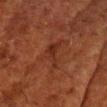| feature | finding |
|---|---|
| biopsy status | no biopsy performed (imaged during a skin exam) |
| diameter | ≈4.5 mm |
| site | the head or neck |
| patient | male, aged approximately 60 |
| image | ~15 mm tile from a whole-body skin photo |
| tile lighting | cross-polarized illumination |